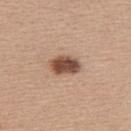  biopsy_status: not biopsied; imaged during a skin examination
  lighting: white-light
  image:
    source: total-body photography crop
    field_of_view_mm: 15
  site: back
  lesion_size:
    long_diameter_mm_approx: 3.5
  patient:
    sex: female
    age_approx: 40
  automated_metrics:
    area_mm2_approx: 7.5
    eccentricity: 0.75
    shape_asymmetry: 0.15
    border_irregularity_0_10: 1.5
    color_variation_0_10: 4.5